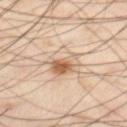Impression:
The lesion was photographed on a routine skin check and not biopsied; there is no pathology result.
Context:
Approximately 5 mm at its widest. The tile uses cross-polarized illumination. From the left thigh. A male patient, in their mid-50s. This image is a 15 mm lesion crop taken from a total-body photograph.